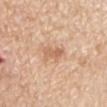Imaged during a routine full-body skin examination; the lesion was not biopsied and no histopathology is available.
The tile uses white-light illumination.
The recorded lesion diameter is about 3 mm.
A male subject aged around 60.
Located on the right upper arm.
Cropped from a whole-body photographic skin survey; the tile spans about 15 mm.